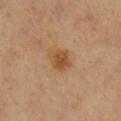Impression: The lesion was tiled from a total-body skin photograph and was not biopsied. Image and clinical context: The subject is a female roughly 70 years of age. Cropped from a whole-body photographic skin survey; the tile spans about 15 mm. About 3 mm across. Imaged with cross-polarized lighting. The lesion is located on the right lower leg.